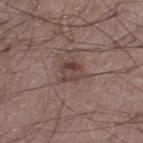Impression: Recorded during total-body skin imaging; not selected for excision or biopsy. Clinical summary: The subject is a male approximately 50 years of age. A 15 mm close-up tile from a total-body photography series done for melanoma screening. On the left thigh.